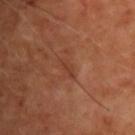Part of a total-body skin-imaging series; this lesion was reviewed on a skin check and was not flagged for biopsy. The subject is a male approximately 55 years of age. The lesion is located on the chest. This image is a 15 mm lesion crop taken from a total-body photograph.